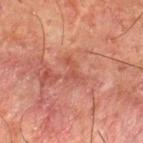- lighting — cross-polarized
- acquisition — total-body-photography crop, ~15 mm field of view
- body site — the upper back
- subject — male, about 50 years old